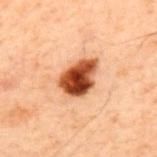| field | value |
|---|---|
| follow-up | no biopsy performed (imaged during a skin exam) |
| tile lighting | cross-polarized illumination |
| imaging modality | 15 mm crop, total-body photography |
| anatomic site | the back |
| patient | male, aged 58 to 62 |